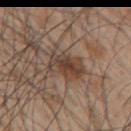  biopsy_status: not biopsied; imaged during a skin examination
  automated_metrics:
    area_mm2_approx: 11.0
    eccentricity: 0.85
    shape_asymmetry: 0.25
    cielab_L: 42
    cielab_a: 15
    cielab_b: 26
    vs_skin_contrast_norm: 8.0
    border_irregularity_0_10: 5.0
    color_variation_0_10: 5.5
    lesion_detection_confidence_0_100: 65
  patient:
    sex: male
    age_approx: 50
  lesion_size:
    long_diameter_mm_approx: 5.5
  site: right upper arm
  image:
    source: total-body photography crop
    field_of_view_mm: 15
  lighting: white-light